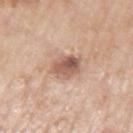Case summary:
• notes — total-body-photography surveillance lesion; no biopsy
• lesion diameter — ~3.5 mm (longest diameter)
• subject — male, approximately 70 years of age
• image — ~15 mm crop, total-body skin-cancer survey
• location — the left upper arm